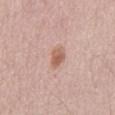Recorded during total-body skin imaging; not selected for excision or biopsy.
A male patient, roughly 65 years of age.
From the abdomen.
Captured under white-light illumination.
Automated image analysis of the tile measured a mean CIELAB color near L≈60 a*≈21 b*≈28, about 11 CIELAB-L* units darker than the surrounding skin, and a normalized lesion–skin contrast near 7.5.
Cropped from a total-body skin-imaging series; the visible field is about 15 mm.